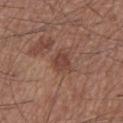Impression:
Imaged during a routine full-body skin examination; the lesion was not biopsied and no histopathology is available.
Context:
From the left lower leg. A male patient aged 58–62. A roughly 15 mm field-of-view crop from a total-body skin photograph.